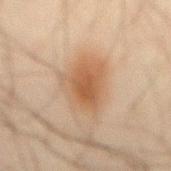The lesion was photographed on a routine skin check and not biopsied; there is no pathology result. The lesion is located on the abdomen. A male patient, roughly 45 years of age. A 15 mm crop from a total-body photograph taken for skin-cancer surveillance. Automated tile analysis of the lesion measured an average lesion color of about L≈47 a*≈16 b*≈29 (CIELAB), a lesion–skin lightness drop of about 8, and a lesion-to-skin contrast of about 7.5 (normalized; higher = more distinct). The analysis additionally found a classifier nevus-likeness of about 95/100. The tile uses cross-polarized illumination. The lesion's longest dimension is about 6.5 mm.